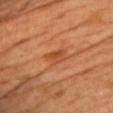Q: Automated lesion metrics?
A: a lesion area of about 3.5 mm², a shape eccentricity near 0.8, and two-axis asymmetry of about 0.4; an average lesion color of about L≈50 a*≈30 b*≈41 (CIELAB)
Q: What are the patient's age and sex?
A: male, aged 38 to 42
Q: How large is the lesion?
A: ~3 mm (longest diameter)
Q: What is the imaging modality?
A: ~15 mm crop, total-body skin-cancer survey
Q: Illumination type?
A: cross-polarized
Q: Lesion location?
A: the chest